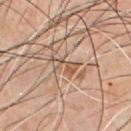Clinical impression:
No biopsy was performed on this lesion — it was imaged during a full skin examination and was not determined to be concerning.
Image and clinical context:
The tile uses cross-polarized illumination. Cropped from a whole-body photographic skin survey; the tile spans about 15 mm. A male patient aged 43–47. From the chest. Automated tile analysis of the lesion measured an average lesion color of about L≈51 a*≈17 b*≈28 (CIELAB), about 8 CIELAB-L* units darker than the surrounding skin, and a normalized lesion–skin contrast near 6.5. The analysis additionally found border irregularity of about 7 on a 0–10 scale and a within-lesion color-variation index near 3/10.